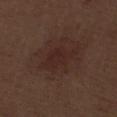Clinical impression:
Part of a total-body skin-imaging series; this lesion was reviewed on a skin check and was not flagged for biopsy.
Context:
From the right thigh. Measured at roughly 5 mm in maximum diameter. An algorithmic analysis of the crop reported a lesion area of about 9 mm², an outline eccentricity of about 0.85 (0 = round, 1 = elongated), and two-axis asymmetry of about 0.25. It also reported a mean CIELAB color near L≈25 a*≈18 b*≈20, roughly 5 lightness units darker than nearby skin, and a normalized lesion–skin contrast near 6. Imaged with white-light lighting. The subject is a male aged around 70. A 15 mm crop from a total-body photograph taken for skin-cancer surveillance.